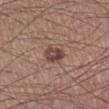Imaged during a routine full-body skin examination; the lesion was not biopsied and no histopathology is available.
Imaged with white-light lighting.
Cropped from a total-body skin-imaging series; the visible field is about 15 mm.
The recorded lesion diameter is about 2.5 mm.
The lesion is on the right lower leg.
The subject is a male aged around 55.